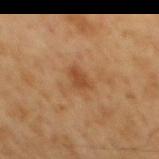Q: Was a biopsy performed?
A: no biopsy performed (imaged during a skin exam)
Q: What is the lesion's diameter?
A: ≈2.5 mm
Q: What kind of image is this?
A: 15 mm crop, total-body photography
Q: Lesion location?
A: the mid back
Q: Who is the patient?
A: male, approximately 60 years of age
Q: What did automated image analysis measure?
A: a border-irregularity index near 2.5/10 and a peripheral color-asymmetry measure near 0.5
Q: What lighting was used for the tile?
A: cross-polarized illumination